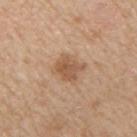Q: Is there a histopathology result?
A: no biopsy performed (imaged during a skin exam)
Q: What lighting was used for the tile?
A: white-light illumination
Q: What kind of image is this?
A: total-body-photography crop, ~15 mm field of view
Q: What did automated image analysis measure?
A: border irregularity of about 3 on a 0–10 scale and a peripheral color-asymmetry measure near 1; a nevus-likeness score of about 40/100 and a lesion-detection confidence of about 100/100
Q: Patient demographics?
A: male, aged 58 to 62
Q: What is the lesion's diameter?
A: ≈3.5 mm
Q: What is the anatomic site?
A: the right upper arm